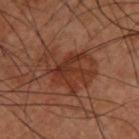Q: Is there a histopathology result?
A: imaged on a skin check; not biopsied
Q: Where on the body is the lesion?
A: the back
Q: What kind of image is this?
A: ~15 mm crop, total-body skin-cancer survey
Q: What lighting was used for the tile?
A: cross-polarized illumination
Q: What did automated image analysis measure?
A: a lesion color around L≈34 a*≈23 b*≈29 in CIELAB and a normalized lesion–skin contrast near 7.5; border irregularity of about 6 on a 0–10 scale and a color-variation rating of about 4.5/10; lesion-presence confidence of about 100/100
Q: What is the lesion's diameter?
A: ≈6 mm
Q: Who is the patient?
A: male, in their 60s